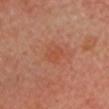biopsy_status: not biopsied; imaged during a skin examination
automated_metrics:
  area_mm2_approx: 4.5
  eccentricity: 0.65
  shape_asymmetry: 0.25
  cielab_L: 51
  cielab_a: 29
  cielab_b: 35
  vs_skin_darker_L: 5.0
  vs_skin_contrast_norm: 4.5
image:
  source: total-body photography crop
  field_of_view_mm: 15
site: chest
lesion_size:
  long_diameter_mm_approx: 2.5
lighting: cross-polarized
patient:
  sex: female
  age_approx: 55